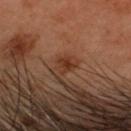Q: Automated lesion metrics?
A: an area of roughly 3.5 mm², an outline eccentricity of about 0.7 (0 = round, 1 = elongated), and a shape-asymmetry score of about 0.35 (0 = symmetric); a mean CIELAB color near L≈27 a*≈19 b*≈25, about 6 CIELAB-L* units darker than the surrounding skin, and a normalized lesion–skin contrast near 7.5; a classifier nevus-likeness of about 35/100
Q: Lesion size?
A: ≈2.5 mm
Q: What kind of image is this?
A: ~15 mm crop, total-body skin-cancer survey
Q: Where on the body is the lesion?
A: the head or neck
Q: Who is the patient?
A: male, aged around 55